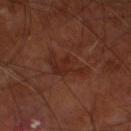Impression:
The lesion was tiled from a total-body skin photograph and was not biopsied.
Acquisition and patient details:
From the left thigh. A male subject roughly 65 years of age. A 15 mm close-up extracted from a 3D total-body photography capture. Approximately 4.5 mm at its widest. The total-body-photography lesion software estimated an automated nevus-likeness rating near 0 out of 100 and a lesion-detection confidence of about 100/100. The tile uses cross-polarized illumination.